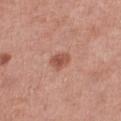Q: Is there a histopathology result?
A: catalogued during a skin exam; not biopsied
Q: Lesion size?
A: ≈2.5 mm
Q: Where on the body is the lesion?
A: the right thigh
Q: What lighting was used for the tile?
A: white-light illumination
Q: What are the patient's age and sex?
A: female, roughly 70 years of age
Q: How was this image acquired?
A: total-body-photography crop, ~15 mm field of view